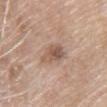The lesion was photographed on a routine skin check and not biopsied; there is no pathology result.
A female patient, aged approximately 75.
The recorded lesion diameter is about 4.5 mm.
The lesion is on the chest.
Cropped from a total-body skin-imaging series; the visible field is about 15 mm.